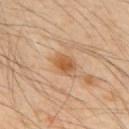  biopsy_status: not biopsied; imaged during a skin examination
  patient:
    sex: male
    age_approx: 50
  lesion_size:
    long_diameter_mm_approx: 3.0
  image:
    source: total-body photography crop
    field_of_view_mm: 15
  site: upper back
  lighting: cross-polarized